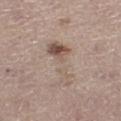Context: The lesion is on the leg. A female patient aged around 50. A 15 mm crop from a total-body photograph taken for skin-cancer surveillance.